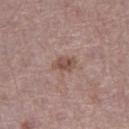The lesion was photographed on a routine skin check and not biopsied; there is no pathology result. The recorded lesion diameter is about 2.5 mm. Captured under white-light illumination. A female subject, about 40 years old. The lesion is located on the left thigh. A region of skin cropped from a whole-body photographic capture, roughly 15 mm wide.